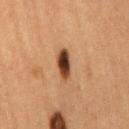Case summary:
* biopsy status · catalogued during a skin exam; not biopsied